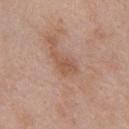The lesion was tiled from a total-body skin photograph and was not biopsied.
Approximately 4 mm at its widest.
Located on the chest.
This is a white-light tile.
The lesion-visualizer software estimated an outline eccentricity of about 0.85 (0 = round, 1 = elongated) and a shape-asymmetry score of about 0.4 (0 = symmetric). It also reported roughly 8 lightness units darker than nearby skin and a normalized lesion–skin contrast near 6. And it measured an automated nevus-likeness rating near 0 out of 100 and a lesion-detection confidence of about 100/100.
A male subject, aged 53–57.
A region of skin cropped from a whole-body photographic capture, roughly 15 mm wide.